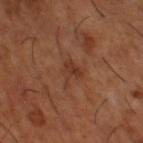Clinical impression: The lesion was tiled from a total-body skin photograph and was not biopsied. Image and clinical context: About 3.5 mm across. A male subject, approximately 65 years of age. Captured under cross-polarized illumination. The lesion is on the right thigh. A 15 mm close-up tile from a total-body photography series done for melanoma screening. Automated image analysis of the tile measured a lesion color around L≈36 a*≈22 b*≈30 in CIELAB and a normalized border contrast of about 6. The software also gave border irregularity of about 5.5 on a 0–10 scale, internal color variation of about 3 on a 0–10 scale, and radial color variation of about 1. It also reported a classifier nevus-likeness of about 0/100.